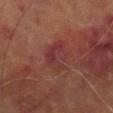Notes:
• follow-up — imaged on a skin check; not biopsied
• image — ~15 mm tile from a whole-body skin photo
• lighting — cross-polarized
• subject — male, in their 70s
• lesion diameter — about 4 mm
• anatomic site — the leg